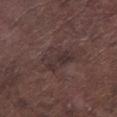Findings:
* notes · no biopsy performed (imaged during a skin exam)
* lesion size · ≈4.5 mm
* image source · ~15 mm crop, total-body skin-cancer survey
* automated lesion analysis · an area of roughly 10 mm², an eccentricity of roughly 0.8, and a shape-asymmetry score of about 0.35 (0 = symmetric); a mean CIELAB color near L≈32 a*≈14 b*≈15, roughly 6 lightness units darker than nearby skin, and a normalized border contrast of about 6; border irregularity of about 4 on a 0–10 scale, a color-variation rating of about 3.5/10, and a peripheral color-asymmetry measure near 1; a nevus-likeness score of about 0/100 and a lesion-detection confidence of about 65/100
* anatomic site · the left lower leg
* subject · male, roughly 75 years of age